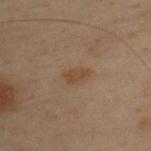Clinical impression:
No biopsy was performed on this lesion — it was imaged during a full skin examination and was not determined to be concerning.
Image and clinical context:
A close-up tile cropped from a whole-body skin photograph, about 15 mm across. Approximately 2.5 mm at its widest. This is a cross-polarized tile. The lesion is located on the back. The subject is a male approximately 50 years of age.